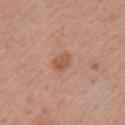{
  "biopsy_status": "not biopsied; imaged during a skin examination",
  "automated_metrics": {
    "area_mm2_approx": 4.5,
    "border_irregularity_0_10": 1.5,
    "color_variation_0_10": 2.5,
    "peripheral_color_asymmetry": 1.0
  },
  "site": "left upper arm",
  "patient": {
    "sex": "female",
    "age_approx": 55
  },
  "lesion_size": {
    "long_diameter_mm_approx": 2.5
  },
  "image": {
    "source": "total-body photography crop",
    "field_of_view_mm": 15
  }
}